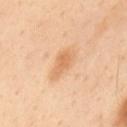Impression: The lesion was photographed on a routine skin check and not biopsied; there is no pathology result. Image and clinical context: The patient is a male in their mid- to late 50s. This is a cross-polarized tile. The lesion's longest dimension is about 3.5 mm. Automated tile analysis of the lesion measured a border-irregularity index near 3/10, a within-lesion color-variation index near 1.5/10, and peripheral color asymmetry of about 0.5. The lesion is located on the upper back. A 15 mm close-up extracted from a 3D total-body photography capture.